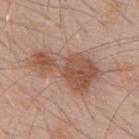<lesion>
  <biopsy_status>not biopsied; imaged during a skin examination</biopsy_status>
  <automated_metrics>
    <area_mm2_approx>19.0</area_mm2_approx>
    <cielab_L>52</cielab_L>
    <cielab_a>21</cielab_a>
    <cielab_b>29</cielab_b>
    <vs_skin_contrast_norm>8.5</vs_skin_contrast_norm>
    <nevus_likeness_0_100>60</nevus_likeness_0_100>
    <lesion_detection_confidence_0_100>100</lesion_detection_confidence_0_100>
  </automated_metrics>
  <site>mid back</site>
  <lighting>white-light</lighting>
  <lesion_size>
    <long_diameter_mm_approx>8.0</long_diameter_mm_approx>
  </lesion_size>
  <patient>
    <sex>male</sex>
    <age_approx>55</age_approx>
  </patient>
  <image>
    <source>total-body photography crop</source>
    <field_of_view_mm>15</field_of_view_mm>
  </image>
</lesion>